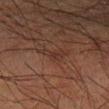Imaged during a routine full-body skin examination; the lesion was not biopsied and no histopathology is available.